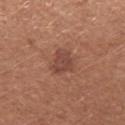notes = catalogued during a skin exam; not biopsied | subject = female, in their mid-30s | anatomic site = the left forearm | image source = total-body-photography crop, ~15 mm field of view.